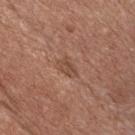Clinical impression:
No biopsy was performed on this lesion — it was imaged during a full skin examination and was not determined to be concerning.
Context:
Longest diameter approximately 2.5 mm. A close-up tile cropped from a whole-body skin photograph, about 15 mm across. On the front of the torso. Imaged with white-light lighting. A male subject, in their mid-50s.